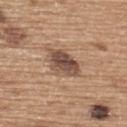Impression:
The lesion was photographed on a routine skin check and not biopsied; there is no pathology result.
Image and clinical context:
Measured at roughly 5 mm in maximum diameter. The lesion is located on the back. A close-up tile cropped from a whole-body skin photograph, about 15 mm across. Captured under white-light illumination. A male patient, aged approximately 65.